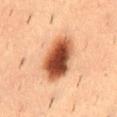Clinical impression: The lesion was photographed on a routine skin check and not biopsied; there is no pathology result. Acquisition and patient details: This image is a 15 mm lesion crop taken from a total-body photograph. Located on the lower back. Automated tile analysis of the lesion measured an area of roughly 18 mm² and a symmetry-axis asymmetry near 0.15. The analysis additionally found a border-irregularity rating of about 1.5/10, a color-variation rating of about 9/10, and radial color variation of about 3. About 6 mm across. A male subject in their mid-50s.